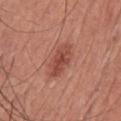follow-up = total-body-photography surveillance lesion; no biopsy | diameter = about 4.5 mm | automated lesion analysis = a mean CIELAB color near L≈48 a*≈28 b*≈29, roughly 10 lightness units darker than nearby skin, and a normalized border contrast of about 7; a border-irregularity rating of about 2/10, a color-variation rating of about 4/10, and radial color variation of about 1.5; a detector confidence of about 100 out of 100 that the crop contains a lesion | site = the chest | subject = male, about 65 years old | acquisition = ~15 mm crop, total-body skin-cancer survey.